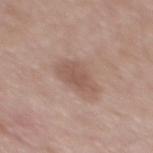workup = catalogued during a skin exam; not biopsied | subject = male, about 55 years old | image = 15 mm crop, total-body photography | site = the mid back | lesion size = ≈4 mm | illumination = white-light illumination | automated metrics = a lesion area of about 9 mm², an eccentricity of roughly 0.75, and two-axis asymmetry of about 0.25; a mean CIELAB color near L≈54 a*≈18 b*≈25, roughly 9 lightness units darker than nearby skin, and a normalized border contrast of about 6.